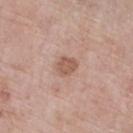Image and clinical context:
From the leg. A lesion tile, about 15 mm wide, cut from a 3D total-body photograph. A male subject, aged approximately 70.